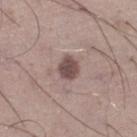Notes:
* follow-up — imaged on a skin check; not biopsied
* image-analysis metrics — an area of roughly 5.5 mm², an outline eccentricity of about 0.45 (0 = round, 1 = elongated), and a symmetry-axis asymmetry near 0.2; border irregularity of about 1.5 on a 0–10 scale and radial color variation of about 0.5; an automated nevus-likeness rating near 55 out of 100
* acquisition — ~15 mm tile from a whole-body skin photo
* patient — male, aged around 50
* lighting — white-light
* body site — the right lower leg